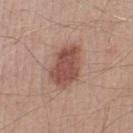Impression: Part of a total-body skin-imaging series; this lesion was reviewed on a skin check and was not flagged for biopsy. Acquisition and patient details: The tile uses white-light illumination. A region of skin cropped from a whole-body photographic capture, roughly 15 mm wide. A male patient, aged around 40. Located on the left lower leg.